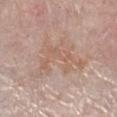Captured during whole-body skin photography for melanoma surveillance; the lesion was not biopsied. A roughly 15 mm field-of-view crop from a total-body skin photograph. A female patient, in their mid- to late 50s. Captured under white-light illumination. From the right lower leg. The lesion-visualizer software estimated a footprint of about 21 mm², an outline eccentricity of about 0.75 (0 = round, 1 = elongated), and two-axis asymmetry of about 0.5. The software also gave an average lesion color of about L≈60 a*≈17 b*≈27 (CIELAB), about 6 CIELAB-L* units darker than the surrounding skin, and a lesion-to-skin contrast of about 4.5 (normalized; higher = more distinct).